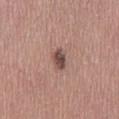The lesion was photographed on a routine skin check and not biopsied; there is no pathology result. The lesion is located on the mid back. A male subject, about 40 years old. Measured at roughly 3 mm in maximum diameter. Cropped from a whole-body photographic skin survey; the tile spans about 15 mm.